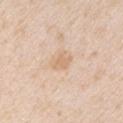The lesion was photographed on a routine skin check and not biopsied; there is no pathology result. The patient is a male approximately 45 years of age. The lesion-visualizer software estimated a lesion color around L≈70 a*≈17 b*≈33 in CIELAB and a lesion-to-skin contrast of about 5 (normalized; higher = more distinct). The analysis additionally found a nevus-likeness score of about 0/100. Cropped from a whole-body photographic skin survey; the tile spans about 15 mm. This is a white-light tile. About 2.5 mm across. The lesion is located on the right upper arm.